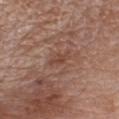notes: imaged on a skin check; not biopsied | lesion size: ≈3.5 mm | subject: male, aged around 80 | site: the chest | image source: ~15 mm crop, total-body skin-cancer survey | tile lighting: white-light illumination.